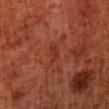Findings:
- biopsy status: total-body-photography surveillance lesion; no biopsy
- lighting: cross-polarized
- patient: male, aged 78 to 82
- site: the right lower leg
- image: ~15 mm tile from a whole-body skin photo
- automated lesion analysis: an area of roughly 3.5 mm², an outline eccentricity of about 0.9 (0 = round, 1 = elongated), and a symmetry-axis asymmetry near 0.4; roughly 4 lightness units darker than nearby skin and a normalized border contrast of about 4.5; a border-irregularity index near 4/10 and a within-lesion color-variation index near 1/10
- lesion size: about 3 mm